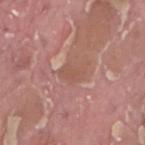Q: Is there a histopathology result?
A: total-body-photography surveillance lesion; no biopsy
Q: What kind of image is this?
A: ~15 mm tile from a whole-body skin photo
Q: Lesion location?
A: the right thigh
Q: Who is the patient?
A: male, roughly 40 years of age